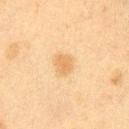Notes:
- follow-up — no biopsy performed (imaged during a skin exam)
- site — the right upper arm
- subject — female, about 40 years old
- acquisition — ~15 mm crop, total-body skin-cancer survey